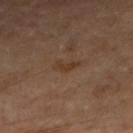No biopsy was performed on this lesion — it was imaged during a full skin examination and was not determined to be concerning. A female subject aged 58 to 62. The lesion-visualizer software estimated a footprint of about 3 mm², an outline eccentricity of about 0.9 (0 = round, 1 = elongated), and two-axis asymmetry of about 0.45. And it measured roughly 6 lightness units darker than nearby skin and a normalized lesion–skin contrast near 6.5. Longest diameter approximately 3 mm. The lesion is located on the right forearm. Cropped from a total-body skin-imaging series; the visible field is about 15 mm. Imaged with cross-polarized lighting.